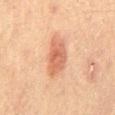follow-up: total-body-photography surveillance lesion; no biopsy | site: the mid back | automated metrics: an automated nevus-likeness rating near 100 out of 100 and lesion-presence confidence of about 100/100 | tile lighting: cross-polarized illumination | subject: male, approximately 70 years of age | image: 15 mm crop, total-body photography.